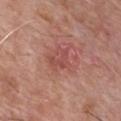Imaged during a routine full-body skin examination; the lesion was not biopsied and no histopathology is available. This is a white-light tile. From the chest. Automated image analysis of the tile measured a border-irregularity index near 7/10 and internal color variation of about 0 on a 0–10 scale. The recorded lesion diameter is about 2.5 mm. The subject is a male about 60 years old. A region of skin cropped from a whole-body photographic capture, roughly 15 mm wide.